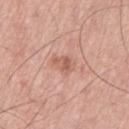workup: imaged on a skin check; not biopsied | image source: ~15 mm crop, total-body skin-cancer survey | subject: male, aged around 70 | diameter: about 2.5 mm | anatomic site: the left thigh | automated metrics: border irregularity of about 2 on a 0–10 scale, a within-lesion color-variation index near 3.5/10, and a peripheral color-asymmetry measure near 1.5.